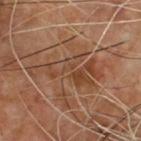Assessment:
Captured during whole-body skin photography for melanoma surveillance; the lesion was not biopsied.
Background:
About 8 mm across. The patient is a male aged around 55. A lesion tile, about 15 mm wide, cut from a 3D total-body photograph. The tile uses cross-polarized illumination. The lesion is on the chest. Automated image analysis of the tile measured a mean CIELAB color near L≈40 a*≈19 b*≈29, a lesion–skin lightness drop of about 7, and a normalized border contrast of about 6. The analysis additionally found a border-irregularity rating of about 9/10, a within-lesion color-variation index near 7/10, and a peripheral color-asymmetry measure near 2.5.